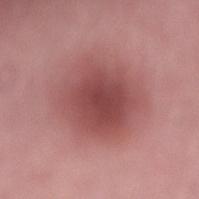Assessment:
Imaged during a routine full-body skin examination; the lesion was not biopsied and no histopathology is available.
Background:
Captured under white-light illumination. The lesion is located on the lower back. Approximately 6.5 mm at its widest. The subject is a male roughly 55 years of age. Cropped from a whole-body photographic skin survey; the tile spans about 15 mm.